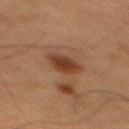follow-up: imaged on a skin check; not biopsied
automated metrics: an area of roughly 6.5 mm², an eccentricity of roughly 0.5, and two-axis asymmetry of about 0.2; roughly 9 lightness units darker than nearby skin and a lesion-to-skin contrast of about 9 (normalized; higher = more distinct); a nevus-likeness score of about 95/100
subject: male, in their 60s
body site: the upper back
tile lighting: cross-polarized
acquisition: 15 mm crop, total-body photography
lesion size: ≈3 mm